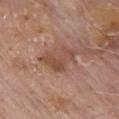Impression: Imaged during a routine full-body skin examination; the lesion was not biopsied and no histopathology is available. Background: A 15 mm close-up tile from a total-body photography series done for melanoma screening. From the chest. The tile uses white-light illumination. A male subject, about 80 years old. About 5 mm across.